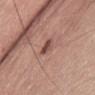automated lesion analysis: a footprint of about 3.5 mm² and an eccentricity of roughly 0.8; a lesion–skin lightness drop of about 12 and a lesion-to-skin contrast of about 8.5 (normalized; higher = more distinct); internal color variation of about 4 on a 0–10 scale
imaging modality: ~15 mm tile from a whole-body skin photo
body site: the abdomen
subject: male, approximately 65 years of age
lighting: white-light